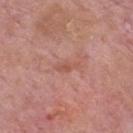This lesion was catalogued during total-body skin photography and was not selected for biopsy. A male patient roughly 75 years of age. A 15 mm crop from a total-body photograph taken for skin-cancer surveillance. From the back.